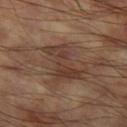| feature | finding |
|---|---|
| biopsy status | total-body-photography surveillance lesion; no biopsy |
| lighting | cross-polarized illumination |
| subject | male, aged 58 to 62 |
| lesion diameter | ≈5.5 mm |
| automated lesion analysis | a lesion color around L≈36 a*≈17 b*≈24 in CIELAB, roughly 8 lightness units darker than nearby skin, and a normalized border contrast of about 7; internal color variation of about 3 on a 0–10 scale and radial color variation of about 1; a nevus-likeness score of about 0/100 |
| location | the left thigh |
| imaging modality | ~15 mm crop, total-body skin-cancer survey |